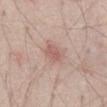Part of a total-body skin-imaging series; this lesion was reviewed on a skin check and was not flagged for biopsy. Located on the chest. A 15 mm close-up tile from a total-body photography series done for melanoma screening. The recorded lesion diameter is about 2.5 mm. The tile uses white-light illumination. The lesion-visualizer software estimated a lesion color around L≈58 a*≈22 b*≈24 in CIELAB and a normalized border contrast of about 6. It also reported a color-variation rating of about 1.5/10 and radial color variation of about 0.5. The software also gave a nevus-likeness score of about 10/100 and lesion-presence confidence of about 100/100. A male patient about 70 years old.